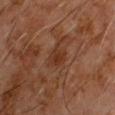{
  "biopsy_status": "not biopsied; imaged during a skin examination",
  "patient": {
    "sex": "male",
    "age_approx": 60
  },
  "image": {
    "source": "total-body photography crop",
    "field_of_view_mm": 15
  },
  "site": "chest"
}